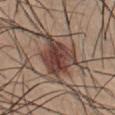The lesion was tiled from a total-body skin photograph and was not biopsied. Imaged with white-light lighting. On the front of the torso. A region of skin cropped from a whole-body photographic capture, roughly 15 mm wide. The patient is a male approximately 20 years of age. Measured at roughly 6.5 mm in maximum diameter.